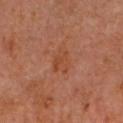The lesion was photographed on a routine skin check and not biopsied; there is no pathology result. From the head or neck. The recorded lesion diameter is about 3 mm. A lesion tile, about 15 mm wide, cut from a 3D total-body photograph. A male subject aged 58 to 62. An algorithmic analysis of the crop reported a lesion area of about 5 mm², an eccentricity of roughly 0.8, and a shape-asymmetry score of about 0.3 (0 = symmetric). And it measured a border-irregularity rating of about 3.5/10, a within-lesion color-variation index near 1.5/10, and a peripheral color-asymmetry measure near 0.5.